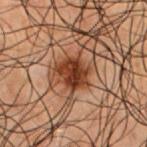The lesion was tiled from a total-body skin photograph and was not biopsied. A 15 mm crop from a total-body photograph taken for skin-cancer surveillance. Located on the chest. The subject is a male aged 48 to 52.